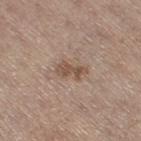biopsy_status: not biopsied; imaged during a skin examination
lighting: white-light
site: right thigh
image:
  source: total-body photography crop
  field_of_view_mm: 15
patient:
  sex: female
  age_approx: 65